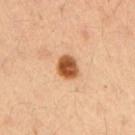The lesion was tiled from a total-body skin photograph and was not biopsied.
The patient is a male aged 38–42.
Longest diameter approximately 3 mm.
A 15 mm close-up extracted from a 3D total-body photography capture.
On the right upper arm.